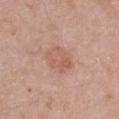follow-up — total-body-photography surveillance lesion; no biopsy | image — total-body-photography crop, ~15 mm field of view | location — the chest | subject — female, approximately 50 years of age.